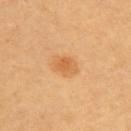follow-up: total-body-photography surveillance lesion; no biopsy
diameter: about 3 mm
image: total-body-photography crop, ~15 mm field of view
anatomic site: the back
automated lesion analysis: an eccentricity of roughly 0.7 and a shape-asymmetry score of about 0.15 (0 = symmetric); an average lesion color of about L≈62 a*≈25 b*≈44 (CIELAB) and a lesion-to-skin contrast of about 6 (normalized; higher = more distinct)
patient: approximately 60 years of age
illumination: cross-polarized